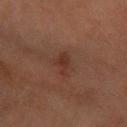The lesion was tiled from a total-body skin photograph and was not biopsied.
A female subject aged 68 to 72.
Measured at roughly 3 mm in maximum diameter.
A lesion tile, about 15 mm wide, cut from a 3D total-body photograph.
From the arm.